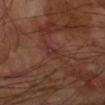This lesion was catalogued during total-body skin photography and was not selected for biopsy.
The recorded lesion diameter is about 2.5 mm.
A male patient, aged 68–72.
A region of skin cropped from a whole-body photographic capture, roughly 15 mm wide.
This is a cross-polarized tile.
The lesion is on the left forearm.